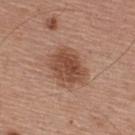biopsy_status: not biopsied; imaged during a skin examination
lesion_size:
  long_diameter_mm_approx: 5.0
image:
  source: total-body photography crop
  field_of_view_mm: 15
site: upper back
patient:
  sex: male
  age_approx: 55
lighting: white-light